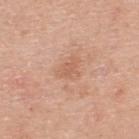This lesion was catalogued during total-body skin photography and was not selected for biopsy.
Captured under white-light illumination.
The lesion is on the upper back.
Measured at roughly 3 mm in maximum diameter.
A female patient aged 28–32.
Automated image analysis of the tile measured an area of roughly 4 mm², a shape eccentricity near 0.7, and a symmetry-axis asymmetry near 0.4. And it measured a mean CIELAB color near L≈61 a*≈22 b*≈33 and a lesion-to-skin contrast of about 5 (normalized; higher = more distinct). And it measured an automated nevus-likeness rating near 0 out of 100.
A lesion tile, about 15 mm wide, cut from a 3D total-body photograph.